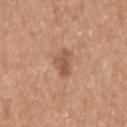follow-up: no biopsy performed (imaged during a skin exam); location: the right upper arm; acquisition: ~15 mm tile from a whole-body skin photo; patient: female, about 50 years old; lesion size: ~2.5 mm (longest diameter).